This is a white-light tile. A lesion tile, about 15 mm wide, cut from a 3D total-body photograph. From the back. A male subject roughly 85 years of age or older:
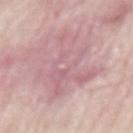diagnosis:
  histopathology: superficial basal cell carcinoma
  malignancy: malignant
  taxonomic_path:
    - Malignant
    - Malignant adnexal epithelial proliferations - Follicular
    - Basal cell carcinoma
    - Basal cell carcinoma, Superficial